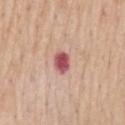Part of a total-body skin-imaging series; this lesion was reviewed on a skin check and was not flagged for biopsy. Located on the back. The patient is a male aged around 70. Approximately 2.5 mm at its widest. The tile uses white-light illumination. An algorithmic analysis of the crop reported a lesion color around L≈53 a*≈31 b*≈21 in CIELAB, a lesion–skin lightness drop of about 18, and a normalized lesion–skin contrast near 12. The software also gave a within-lesion color-variation index near 2/10 and radial color variation of about 0.5. And it measured a classifier nevus-likeness of about 0/100 and lesion-presence confidence of about 100/100. Cropped from a total-body skin-imaging series; the visible field is about 15 mm.